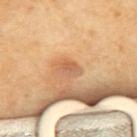Assessment: Captured during whole-body skin photography for melanoma surveillance; the lesion was not biopsied. Context: A region of skin cropped from a whole-body photographic capture, roughly 15 mm wide. The total-body-photography lesion software estimated a footprint of about 4.5 mm², a shape eccentricity near 0.8, and two-axis asymmetry of about 0.15. It also reported lesion-presence confidence of about 100/100. On the upper back. Captured under cross-polarized illumination. The subject is aged 68 to 72. Approximately 3 mm at its widest.